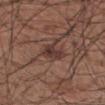lesion size — about 3.5 mm; tile lighting — white-light; patient — male, aged 53–57; location — the left forearm; imaging modality — ~15 mm crop, total-body skin-cancer survey.